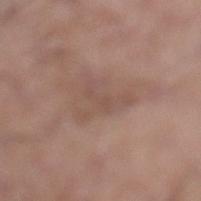Captured during whole-body skin photography for melanoma surveillance; the lesion was not biopsied. The patient is a male aged approximately 60. About 4 mm across. Located on the left lower leg. The lesion-visualizer software estimated a border-irregularity rating of about 9.5/10, internal color variation of about 0.5 on a 0–10 scale, and a peripheral color-asymmetry measure near 0. Captured under white-light illumination. A lesion tile, about 15 mm wide, cut from a 3D total-body photograph.